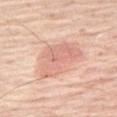Impression: The lesion was photographed on a routine skin check and not biopsied; there is no pathology result. Background: The recorded lesion diameter is about 5.5 mm. The lesion-visualizer software estimated a footprint of about 14 mm², an eccentricity of roughly 0.75, and a shape-asymmetry score of about 0.45 (0 = symmetric). The software also gave a lesion color around L≈69 a*≈24 b*≈29 in CIELAB, about 9 CIELAB-L* units darker than the surrounding skin, and a normalized border contrast of about 5. This is a white-light tile. A 15 mm close-up tile from a total-body photography series done for melanoma screening. The lesion is located on the right thigh. A male subject, aged approximately 80.